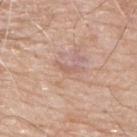biopsy status: catalogued during a skin exam; not biopsied
location: the back
image source: ~15 mm tile from a whole-body skin photo
lesion diameter: about 2 mm
patient: male, approximately 65 years of age